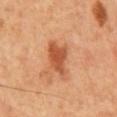Q: Was this lesion biopsied?
A: imaged on a skin check; not biopsied
Q: How large is the lesion?
A: about 5 mm
Q: Who is the patient?
A: male, aged 58–62
Q: What lighting was used for the tile?
A: cross-polarized illumination
Q: What kind of image is this?
A: 15 mm crop, total-body photography
Q: What did automated image analysis measure?
A: a lesion area of about 9.5 mm², a shape eccentricity near 0.85, and a shape-asymmetry score of about 0.35 (0 = symmetric); a mean CIELAB color near L≈48 a*≈26 b*≈35, about 11 CIELAB-L* units darker than the surrounding skin, and a lesion-to-skin contrast of about 8 (normalized; higher = more distinct); an automated nevus-likeness rating near 75 out of 100 and a detector confidence of about 100 out of 100 that the crop contains a lesion
Q: What is the anatomic site?
A: the chest